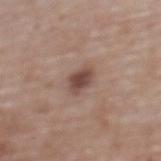No biopsy was performed on this lesion — it was imaged during a full skin examination and was not determined to be concerning. On the back. Imaged with white-light lighting. The total-body-photography lesion software estimated a mean CIELAB color near L≈46 a*≈18 b*≈23, a lesion–skin lightness drop of about 12, and a normalized lesion–skin contrast near 9. The software also gave a nevus-likeness score of about 90/100 and lesion-presence confidence of about 100/100. Approximately 2.5 mm at its widest. Cropped from a whole-body photographic skin survey; the tile spans about 15 mm. A female patient, aged 63–67.